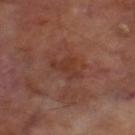Clinical impression: Recorded during total-body skin imaging; not selected for excision or biopsy. Context: The lesion's longest dimension is about 3 mm. A male subject, in their 70s. The lesion-visualizer software estimated a lesion area of about 5.5 mm², a shape eccentricity near 0.55, and a symmetry-axis asymmetry near 0.55. And it measured a border-irregularity rating of about 6/10, a color-variation rating of about 1/10, and a peripheral color-asymmetry measure near 0.5. It also reported a nevus-likeness score of about 0/100 and lesion-presence confidence of about 100/100. The tile uses cross-polarized illumination. A roughly 15 mm field-of-view crop from a total-body skin photograph. From the left thigh.